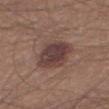Imaged during a routine full-body skin examination; the lesion was not biopsied and no histopathology is available. This image is a 15 mm lesion crop taken from a total-body photograph. A male patient, roughly 25 years of age. Imaged with white-light lighting. The lesion is on the right thigh.